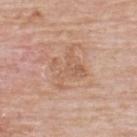Recorded during total-body skin imaging; not selected for excision or biopsy. A close-up tile cropped from a whole-body skin photograph, about 15 mm across. Automated image analysis of the tile measured a symmetry-axis asymmetry near 0.45. It also reported a border-irregularity index near 8.5/10, a color-variation rating of about 3/10, and a peripheral color-asymmetry measure near 1.5. The analysis additionally found a nevus-likeness score of about 0/100. The lesion's longest dimension is about 5 mm. This is a white-light tile. A male patient, aged approximately 75. The lesion is located on the upper back.